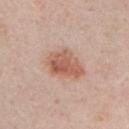biopsy status: no biopsy performed (imaged during a skin exam)
location: the chest
image: ~15 mm crop, total-body skin-cancer survey
subject: male, approximately 50 years of age